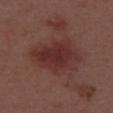biopsy status = imaged on a skin check; not biopsied | site = the upper back | acquisition = 15 mm crop, total-body photography | patient = male, aged approximately 55.